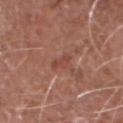This lesion was catalogued during total-body skin photography and was not selected for biopsy.
This image is a 15 mm lesion crop taken from a total-body photograph.
A male subject aged 73–77.
The lesion-visualizer software estimated a lesion area of about 2.5 mm², an eccentricity of roughly 0.9, and a shape-asymmetry score of about 0.35 (0 = symmetric). The software also gave a nevus-likeness score of about 0/100 and a lesion-detection confidence of about 60/100.
On the head or neck.
The recorded lesion diameter is about 3 mm.
Imaged with white-light lighting.